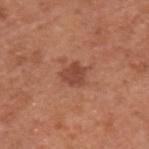Assessment:
No biopsy was performed on this lesion — it was imaged during a full skin examination and was not determined to be concerning.
Background:
Approximately 3 mm at its widest. Located on the upper back. Automated image analysis of the tile measured an average lesion color of about L≈46 a*≈25 b*≈31 (CIELAB), roughly 10 lightness units darker than nearby skin, and a normalized border contrast of about 7. A male patient, aged around 55. A close-up tile cropped from a whole-body skin photograph, about 15 mm across.